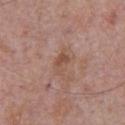diameter: ~3 mm (longest diameter) | body site: the chest | subject: male, about 75 years old | image: ~15 mm crop, total-body skin-cancer survey | image-analysis metrics: a footprint of about 4 mm² and two-axis asymmetry of about 0.25; a mean CIELAB color near L≈51 a*≈20 b*≈28, about 7 CIELAB-L* units darker than the surrounding skin, and a lesion-to-skin contrast of about 6 (normalized; higher = more distinct) | tile lighting: white-light.